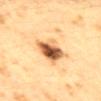Imaged during a routine full-body skin examination; the lesion was not biopsied and no histopathology is available. The subject is a female about 40 years old. Longest diameter approximately 4 mm. A region of skin cropped from a whole-body photographic capture, roughly 15 mm wide. Automated tile analysis of the lesion measured about 18 CIELAB-L* units darker than the surrounding skin and a lesion-to-skin contrast of about 12 (normalized; higher = more distinct). The software also gave internal color variation of about 10 on a 0–10 scale and a peripheral color-asymmetry measure near 3. The software also gave a nevus-likeness score of about 95/100 and a detector confidence of about 100 out of 100 that the crop contains a lesion. This is a cross-polarized tile. The lesion is located on the back.